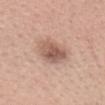{"biopsy_status": "not biopsied; imaged during a skin examination", "image": {"source": "total-body photography crop", "field_of_view_mm": 15}, "patient": {"sex": "female", "age_approx": 40}, "site": "head or neck"}